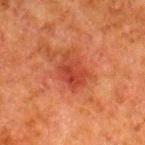Clinical impression:
Captured during whole-body skin photography for melanoma surveillance; the lesion was not biopsied.
Clinical summary:
A male subject, about 80 years old. From the right lower leg. Longest diameter approximately 4 mm. Imaged with cross-polarized lighting. A region of skin cropped from a whole-body photographic capture, roughly 15 mm wide.